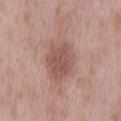No biopsy was performed on this lesion — it was imaged during a full skin examination and was not determined to be concerning. From the back. The subject is a male aged around 55. A 15 mm close-up tile from a total-body photography series done for melanoma screening.